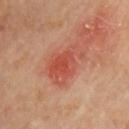Q: Was this lesion biopsied?
A: catalogued during a skin exam; not biopsied
Q: What did automated image analysis measure?
A: a lesion area of about 13 mm², an outline eccentricity of about 0.8 (0 = round, 1 = elongated), and two-axis asymmetry of about 0.35; a border-irregularity index near 4/10, a color-variation rating of about 4.5/10, and radial color variation of about 1.5
Q: How was this image acquired?
A: ~15 mm crop, total-body skin-cancer survey
Q: Patient demographics?
A: male, roughly 60 years of age
Q: What is the anatomic site?
A: the chest